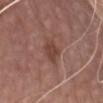Impression:
The lesion was photographed on a routine skin check and not biopsied; there is no pathology result.
Acquisition and patient details:
A roughly 15 mm field-of-view crop from a total-body skin photograph. An algorithmic analysis of the crop reported a classifier nevus-likeness of about 20/100 and lesion-presence confidence of about 100/100. A male subject aged approximately 75. From the front of the torso.